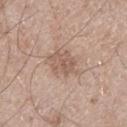Recorded during total-body skin imaging; not selected for excision or biopsy.
This image is a 15 mm lesion crop taken from a total-body photograph.
Automated image analysis of the tile measured border irregularity of about 3.5 on a 0–10 scale, a within-lesion color-variation index near 3/10, and a peripheral color-asymmetry measure near 1.
From the left thigh.
The patient is a male about 65 years old.